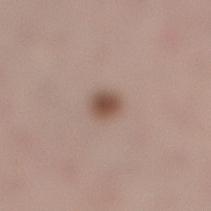The lesion was tiled from a total-body skin photograph and was not biopsied. Automated tile analysis of the lesion measured a footprint of about 4.5 mm² and an outline eccentricity of about 0.6 (0 = round, 1 = elongated). And it measured a mean CIELAB color near L≈51 a*≈17 b*≈25, about 12 CIELAB-L* units darker than the surrounding skin, and a lesion-to-skin contrast of about 9 (normalized; higher = more distinct). It also reported a classifier nevus-likeness of about 100/100 and a detector confidence of about 100 out of 100 that the crop contains a lesion. Located on the right lower leg. A 15 mm close-up tile from a total-body photography series done for melanoma screening. A female subject aged around 25. Measured at roughly 2.5 mm in maximum diameter.